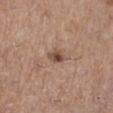This lesion was catalogued during total-body skin photography and was not selected for biopsy. Captured under white-light illumination. The lesion is located on the right lower leg. A female subject, aged around 45. A region of skin cropped from a whole-body photographic capture, roughly 15 mm wide.